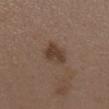workup — imaged on a skin check; not biopsied
body site — the back
image source — ~15 mm tile from a whole-body skin photo
subject — female, roughly 35 years of age
size — ~4 mm (longest diameter)